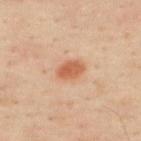Cropped from a whole-body photographic skin survey; the tile spans about 15 mm.
A male patient aged approximately 50.
The lesion is located on the upper back.